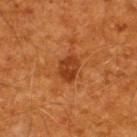  biopsy_status: not biopsied; imaged during a skin examination
  lighting: cross-polarized
  site: back
  patient:
    sex: male
    age_approx: 60
  image:
    source: total-body photography crop
    field_of_view_mm: 15